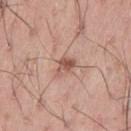Assessment: Imaged during a routine full-body skin examination; the lesion was not biopsied and no histopathology is available. Clinical summary: A region of skin cropped from a whole-body photographic capture, roughly 15 mm wide. Approximately 3 mm at its widest. The subject is a male aged approximately 50. This is a white-light tile. An algorithmic analysis of the crop reported a lesion area of about 4 mm², an outline eccentricity of about 0.75 (0 = round, 1 = elongated), and two-axis asymmetry of about 0.4. On the left thigh.